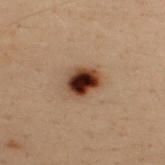The lesion was tiled from a total-body skin photograph and was not biopsied.